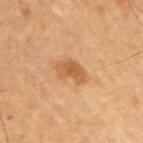  biopsy_status: not biopsied; imaged during a skin examination
  automated_metrics:
    area_mm2_approx: 6.0
    eccentricity: 0.8
    shape_asymmetry: 0.3
    cielab_L: 56
    cielab_a: 23
    cielab_b: 40
    border_irregularity_0_10: 3.5
    color_variation_0_10: 2.5
    peripheral_color_asymmetry: 0.5
    nevus_likeness_0_100: 75
    lesion_detection_confidence_0_100: 100
  image:
    source: total-body photography crop
    field_of_view_mm: 15
  site: right upper arm
  lesion_size:
    long_diameter_mm_approx: 4.0
  patient:
    sex: male
    age_approx: 60
  lighting: cross-polarized